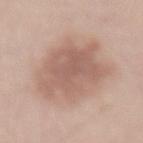This lesion was catalogued during total-body skin photography and was not selected for biopsy. The lesion is on the lower back. A 15 mm close-up tile from a total-body photography series done for melanoma screening. A male subject, aged around 65.